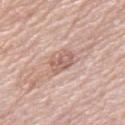Part of a total-body skin-imaging series; this lesion was reviewed on a skin check and was not flagged for biopsy. The tile uses white-light illumination. Measured at roughly 3 mm in maximum diameter. A lesion tile, about 15 mm wide, cut from a 3D total-body photograph. An algorithmic analysis of the crop reported a footprint of about 5.5 mm², an eccentricity of roughly 0.7, and a shape-asymmetry score of about 0.25 (0 = symmetric). It also reported an average lesion color of about L≈60 a*≈20 b*≈25 (CIELAB) and a lesion–skin lightness drop of about 10. And it measured a nevus-likeness score of about 0/100 and a lesion-detection confidence of about 100/100. From the leg. The subject is a female aged approximately 65.